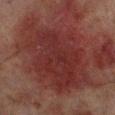Q: Is there a histopathology result?
A: total-body-photography surveillance lesion; no biopsy
Q: Illumination type?
A: cross-polarized
Q: What kind of image is this?
A: total-body-photography crop, ~15 mm field of view
Q: What did automated image analysis measure?
A: a footprint of about 55 mm², a shape eccentricity near 0.75, and two-axis asymmetry of about 0.25; a mean CIELAB color near L≈25 a*≈22 b*≈19, roughly 7 lightness units darker than nearby skin, and a normalized lesion–skin contrast near 7.5; a classifier nevus-likeness of about 0/100
Q: What is the lesion's diameter?
A: about 11 mm
Q: Lesion location?
A: the right lower leg
Q: What are the patient's age and sex?
A: male, aged approximately 70